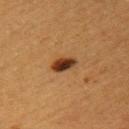  biopsy_status: not biopsied; imaged during a skin examination
  patient:
    sex: female
    age_approx: 40
  lesion_size:
    long_diameter_mm_approx: 3.0
  image:
    source: total-body photography crop
    field_of_view_mm: 15
  automated_metrics:
    area_mm2_approx: 5.5
    eccentricity: 0.75
    shape_asymmetry: 0.15
    border_irregularity_0_10: 1.5
    color_variation_0_10: 5.5
    peripheral_color_asymmetry: 1.5
    nevus_likeness_0_100: 100
  site: left upper arm